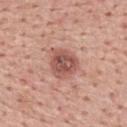Clinical impression: Captured during whole-body skin photography for melanoma surveillance; the lesion was not biopsied. Acquisition and patient details: The lesion-visualizer software estimated a lesion area of about 10 mm² and an outline eccentricity of about 0.35 (0 = round, 1 = elongated). It also reported an average lesion color of about L≈53 a*≈24 b*≈26 (CIELAB), about 12 CIELAB-L* units darker than the surrounding skin, and a normalized border contrast of about 8.5. The analysis additionally found a border-irregularity index near 1.5/10 and a within-lesion color-variation index near 5/10. This is a white-light tile. Measured at roughly 4 mm in maximum diameter. The patient is a male about 60 years old. A lesion tile, about 15 mm wide, cut from a 3D total-body photograph. The lesion is located on the upper back.